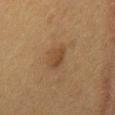The lesion was photographed on a routine skin check and not biopsied; there is no pathology result. This is a cross-polarized tile. The recorded lesion diameter is about 3 mm. Located on the mid back. A female patient aged 48 to 52. Cropped from a whole-body photographic skin survey; the tile spans about 15 mm.